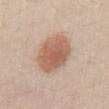lesion diameter: about 5.5 mm
automated metrics: an area of roughly 19 mm², an outline eccentricity of about 0.55 (0 = round, 1 = elongated), and a symmetry-axis asymmetry near 0.1; roughly 12 lightness units darker than nearby skin and a lesion-to-skin contrast of about 8 (normalized; higher = more distinct)
anatomic site: the abdomen
acquisition: ~15 mm tile from a whole-body skin photo
subject: male, aged around 25
lighting: white-light illumination